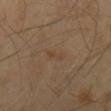The lesion was photographed on a routine skin check and not biopsied; there is no pathology result. A roughly 15 mm field-of-view crop from a total-body skin photograph. Automated tile analysis of the lesion measured a border-irregularity rating of about 4.5/10, a color-variation rating of about 0/10, and peripheral color asymmetry of about 0. It also reported lesion-presence confidence of about 100/100. From the mid back. The subject is a male aged around 40. The lesion's longest dimension is about 2.5 mm. Captured under cross-polarized illumination.